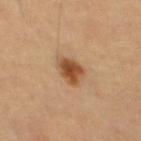Approximately 3 mm at its widest.
A male subject, in their mid- to late 60s.
Captured under cross-polarized illumination.
A close-up tile cropped from a whole-body skin photograph, about 15 mm across.
The lesion is located on the mid back.
Automated image analysis of the tile measured an area of roughly 6.5 mm², an eccentricity of roughly 0.6, and two-axis asymmetry of about 0.25. It also reported a border-irregularity rating of about 2/10 and peripheral color asymmetry of about 1.